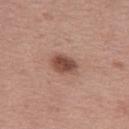Impression:
The lesion was tiled from a total-body skin photograph and was not biopsied.
Clinical summary:
Located on the left thigh. Imaged with white-light lighting. Automated tile analysis of the lesion measured an area of roughly 6.5 mm², an eccentricity of roughly 0.75, and a shape-asymmetry score of about 0.2 (0 = symmetric). The software also gave border irregularity of about 2 on a 0–10 scale and internal color variation of about 4 on a 0–10 scale. The subject is a female roughly 50 years of age. About 3.5 mm across. Cropped from a total-body skin-imaging series; the visible field is about 15 mm.